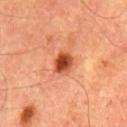acquisition: ~15 mm crop, total-body skin-cancer survey | body site: the chest | patient: male, aged 63–67.